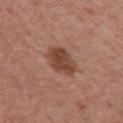workup — total-body-photography surveillance lesion; no biopsy
body site — the upper back
image — 15 mm crop, total-body photography
diameter — ~4.5 mm (longest diameter)
patient — male, aged 43–47
lighting — white-light
automated lesion analysis — an eccentricity of roughly 0.75 and a symmetry-axis asymmetry near 0.2; about 11 CIELAB-L* units darker than the surrounding skin and a normalized border contrast of about 8; border irregularity of about 2 on a 0–10 scale